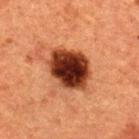workup: catalogued during a skin exam; not biopsied
size: about 5.5 mm
tile lighting: cross-polarized illumination
acquisition: ~15 mm tile from a whole-body skin photo
automated metrics: an average lesion color of about L≈28 a*≈24 b*≈28 (CIELAB), about 21 CIELAB-L* units darker than the surrounding skin, and a normalized border contrast of about 18
subject: male, aged approximately 50
anatomic site: the upper back